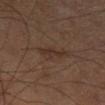{"biopsy_status": "not biopsied; imaged during a skin examination", "image": {"source": "total-body photography crop", "field_of_view_mm": 15}, "patient": {"sex": "male", "age_approx": 60}, "site": "leg", "lesion_size": {"long_diameter_mm_approx": 4.0}, "lighting": "cross-polarized"}